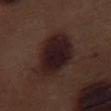Assessment: The lesion was tiled from a total-body skin photograph and was not biopsied. Acquisition and patient details: A roughly 15 mm field-of-view crop from a total-body skin photograph. A male subject, about 70 years old. The tile uses white-light illumination. On the right thigh. About 7.5 mm across.